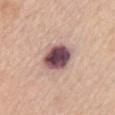Findings:
• subject — female, in their 70s
• diameter — ~4.5 mm (longest diameter)
• anatomic site — the mid back
• lighting — white-light
• image-analysis metrics — a lesion area of about 12 mm², a shape eccentricity near 0.6, and a symmetry-axis asymmetry near 0.1; a lesion–skin lightness drop of about 21 and a normalized border contrast of about 15; a color-variation rating of about 8.5/10 and peripheral color asymmetry of about 2.5; a classifier nevus-likeness of about 30/100 and lesion-presence confidence of about 100/100
• acquisition — total-body-photography crop, ~15 mm field of view